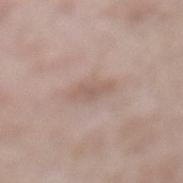workup: no biopsy performed (imaged during a skin exam)